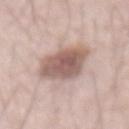{"biopsy_status": "not biopsied; imaged during a skin examination", "site": "abdomen", "lesion_size": {"long_diameter_mm_approx": 5.5}, "image": {"source": "total-body photography crop", "field_of_view_mm": 15}, "patient": {"sex": "male", "age_approx": 70}, "lighting": "white-light"}